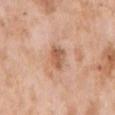notes: no biopsy performed (imaged during a skin exam); image: total-body-photography crop, ~15 mm field of view; patient: female, approximately 70 years of age; tile lighting: white-light illumination; body site: the right upper arm; size: about 2.5 mm.